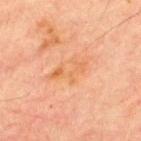Recorded during total-body skin imaging; not selected for excision or biopsy.
This is a cross-polarized tile.
The lesion-visualizer software estimated an eccentricity of roughly 0.85 and two-axis asymmetry of about 0.35. The analysis additionally found an average lesion color of about L≈55 a*≈22 b*≈35 (CIELAB) and a normalized border contrast of about 5.5.
The lesion is on the chest.
A 15 mm close-up extracted from a 3D total-body photography capture.
A male patient, about 70 years old.
The recorded lesion diameter is about 5 mm.